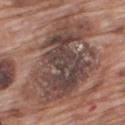<lesion>
<biopsy_status>not biopsied; imaged during a skin examination</biopsy_status>
<site>upper back</site>
<lighting>white-light</lighting>
<image>
  <source>total-body photography crop</source>
  <field_of_view_mm>15</field_of_view_mm>
</image>
<patient>
  <sex>male</sex>
  <age_approx>70</age_approx>
</patient>
<lesion_size>
  <long_diameter_mm_approx>10.5</long_diameter_mm_approx>
</lesion_size>
</lesion>